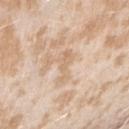workup — no biopsy performed (imaged during a skin exam) | image — ~15 mm crop, total-body skin-cancer survey | site — the arm | illumination — white-light illumination | patient — female, aged 23 to 27.